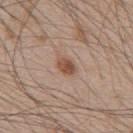anatomic site: the mid back | size: ≈2.5 mm | lighting: white-light | automated metrics: a lesion color around L≈51 a*≈19 b*≈29 in CIELAB, a lesion–skin lightness drop of about 11, and a normalized lesion–skin contrast near 8.5; a border-irregularity rating of about 1.5/10, internal color variation of about 4 on a 0–10 scale, and a peripheral color-asymmetry measure near 1.5; a detector confidence of about 100 out of 100 that the crop contains a lesion | acquisition: 15 mm crop, total-body photography | patient: male, roughly 50 years of age.